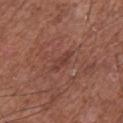workup = no biopsy performed (imaged during a skin exam)
image source = ~15 mm tile from a whole-body skin photo
location = the chest
lighting = white-light
lesion size = ~3 mm (longest diameter)
subject = male, aged approximately 75